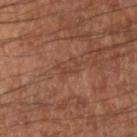Clinical impression:
Captured during whole-body skin photography for melanoma surveillance; the lesion was not biopsied.
Acquisition and patient details:
The tile uses cross-polarized illumination. Automated image analysis of the tile measured a footprint of about 3 mm², a shape eccentricity near 0.75, and two-axis asymmetry of about 0.65. The analysis additionally found a lesion color around L≈40 a*≈21 b*≈28 in CIELAB, roughly 6 lightness units darker than nearby skin, and a normalized lesion–skin contrast near 5. It also reported border irregularity of about 9 on a 0–10 scale and radial color variation of about 0. A roughly 15 mm field-of-view crop from a total-body skin photograph. The lesion's longest dimension is about 2.5 mm. A male subject, roughly 65 years of age. The lesion is on the left arm.